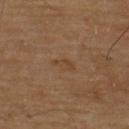Recorded during total-body skin imaging; not selected for excision or biopsy. The lesion's longest dimension is about 2.5 mm. The patient is a male approximately 70 years of age. A close-up tile cropped from a whole-body skin photograph, about 15 mm across. The lesion is on the back. An algorithmic analysis of the crop reported a footprint of about 3 mm², an outline eccentricity of about 0.85 (0 = round, 1 = elongated), and a shape-asymmetry score of about 0.4 (0 = symmetric). It also reported a detector confidence of about 100 out of 100 that the crop contains a lesion.